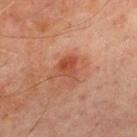{"biopsy_status": "not biopsied; imaged during a skin examination", "image": {"source": "total-body photography crop", "field_of_view_mm": 15}, "patient": {"sex": "male", "age_approx": 70}, "lighting": "cross-polarized", "site": "chest", "lesion_size": {"long_diameter_mm_approx": 3.0}, "automated_metrics": {"eccentricity": 0.55, "shape_asymmetry": 0.35, "nevus_likeness_0_100": 80, "lesion_detection_confidence_0_100": 100}}